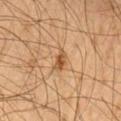Impression: The lesion was photographed on a routine skin check and not biopsied; there is no pathology result. Acquisition and patient details: A male subject, about 65 years old. A close-up tile cropped from a whole-body skin photograph, about 15 mm across. On the left thigh.